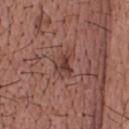– follow-up — catalogued during a skin exam; not biopsied
– anatomic site — the head or neck
– image — 15 mm crop, total-body photography
– patient — male, in their mid-50s
– lesion size — ≈3 mm
– tile lighting — white-light illumination
– image-analysis metrics — a lesion color around L≈40 a*≈22 b*≈24 in CIELAB, about 9 CIELAB-L* units darker than the surrounding skin, and a normalized lesion–skin contrast near 7; a nevus-likeness score of about 25/100 and lesion-presence confidence of about 100/100